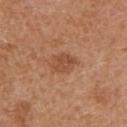Imaged during a routine full-body skin examination; the lesion was not biopsied and no histopathology is available.
A female subject, in their 40s.
From the left upper arm.
A region of skin cropped from a whole-body photographic capture, roughly 15 mm wide.